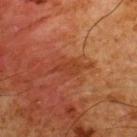Case summary:
- workup · no biopsy performed (imaged during a skin exam)
- imaging modality · total-body-photography crop, ~15 mm field of view
- lesion size · ~3 mm (longest diameter)
- subject · male, about 60 years old
- image-analysis metrics · a lesion area of about 3.5 mm² and an eccentricity of roughly 0.85; a lesion–skin lightness drop of about 5 and a lesion-to-skin contrast of about 5.5 (normalized; higher = more distinct); a classifier nevus-likeness of about 0/100 and lesion-presence confidence of about 85/100
- illumination · cross-polarized
- anatomic site · the upper back